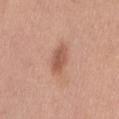Q: How large is the lesion?
A: ≈4 mm
Q: What are the patient's age and sex?
A: male, aged around 30
Q: What is the imaging modality?
A: total-body-photography crop, ~15 mm field of view
Q: What is the anatomic site?
A: the mid back
Q: Automated lesion metrics?
A: a lesion color around L≈56 a*≈23 b*≈30 in CIELAB, about 11 CIELAB-L* units darker than the surrounding skin, and a normalized lesion–skin contrast near 7; a border-irregularity index near 2/10 and a peripheral color-asymmetry measure near 1